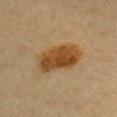workup: catalogued during a skin exam; not biopsied
subject: female, aged 38–42
acquisition: total-body-photography crop, ~15 mm field of view
anatomic site: the chest
automated metrics: a footprint of about 17 mm², a shape eccentricity near 0.85, and a shape-asymmetry score of about 0.15 (0 = symmetric); a border-irregularity rating of about 2/10, a color-variation rating of about 4.5/10, and peripheral color asymmetry of about 1.5; a classifier nevus-likeness of about 100/100 and a detector confidence of about 100 out of 100 that the crop contains a lesion
lesion diameter: about 6 mm
lighting: cross-polarized